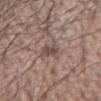biopsy status = catalogued during a skin exam; not biopsied
acquisition = 15 mm crop, total-body photography
patient = male, aged 53–57
site = the arm
tile lighting = white-light illumination
image-analysis metrics = an average lesion color of about L≈47 a*≈16 b*≈21 (CIELAB) and a lesion–skin lightness drop of about 9; a detector confidence of about 100 out of 100 that the crop contains a lesion
diameter = about 3 mm